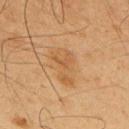<lesion>
  <biopsy_status>not biopsied; imaged during a skin examination</biopsy_status>
  <lesion_size>
    <long_diameter_mm_approx>5.0</long_diameter_mm_approx>
  </lesion_size>
  <patient>
    <sex>male</sex>
    <age_approx>65</age_approx>
  </patient>
  <image>
    <source>total-body photography crop</source>
    <field_of_view_mm>15</field_of_view_mm>
  </image>
  <lighting>cross-polarized</lighting>
  <site>upper back</site>
</lesion>